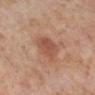A close-up tile cropped from a whole-body skin photograph, about 15 mm across.
A female subject, aged 53–57.
The lesion is on the right lower leg.